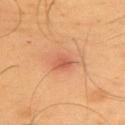Clinical impression:
This lesion was catalogued during total-body skin photography and was not selected for biopsy.
Context:
Automated image analysis of the tile measured roughly 9 lightness units darker than nearby skin and a lesion-to-skin contrast of about 6.5 (normalized; higher = more distinct). The analysis additionally found a border-irregularity index near 2/10 and a within-lesion color-variation index near 2.5/10. The analysis additionally found a nevus-likeness score of about 0/100. This is a cross-polarized tile. The recorded lesion diameter is about 3 mm. A close-up tile cropped from a whole-body skin photograph, about 15 mm across. A male subject approximately 55 years of age. The lesion is on the upper back.